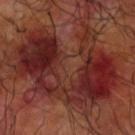Q: Is there a histopathology result?
A: catalogued during a skin exam; not biopsied
Q: How was this image acquired?
A: 15 mm crop, total-body photography
Q: What is the anatomic site?
A: the right upper arm
Q: Who is the patient?
A: male, roughly 60 years of age